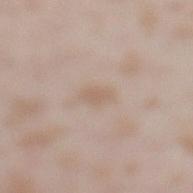Impression:
The lesion was tiled from a total-body skin photograph and was not biopsied.
Background:
A female subject aged 23–27. A 15 mm close-up extracted from a 3D total-body photography capture. From the leg. An algorithmic analysis of the crop reported a lesion color around L≈61 a*≈14 b*≈27 in CIELAB and a lesion-to-skin contrast of about 5 (normalized; higher = more distinct). It also reported a classifier nevus-likeness of about 0/100 and a lesion-detection confidence of about 100/100. The tile uses white-light illumination.